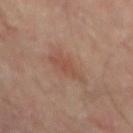patient — male, approximately 65 years of age; image — 15 mm crop, total-body photography; site — the back; diameter — ≈4 mm.